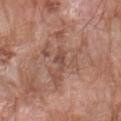subject — male, about 60 years old
size — about 2.5 mm
anatomic site — the arm
acquisition — ~15 mm crop, total-body skin-cancer survey
illumination — white-light illumination